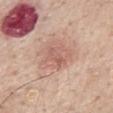Notes:
– follow-up: imaged on a skin check; not biopsied
– imaging modality: ~15 mm crop, total-body skin-cancer survey
– lesion size: about 3.5 mm
– automated lesion analysis: an average lesion color of about L≈59 a*≈22 b*≈26 (CIELAB) and a lesion–skin lightness drop of about 7; a classifier nevus-likeness of about 0/100 and a detector confidence of about 100 out of 100 that the crop contains a lesion
– location: the mid back
– patient: male, approximately 55 years of age
– illumination: white-light illumination